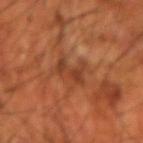  biopsy_status: not biopsied; imaged during a skin examination
  site: left forearm
  patient:
    sex: male
    age_approx: 70
  lesion_size:
    long_diameter_mm_approx: 3.0
  image:
    source: total-body photography crop
    field_of_view_mm: 15
  lighting: cross-polarized